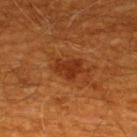Q: Was this lesion biopsied?
A: total-body-photography surveillance lesion; no biopsy
Q: Illumination type?
A: cross-polarized
Q: What is the lesion's diameter?
A: ≈3.5 mm
Q: Lesion location?
A: the upper back
Q: Who is the patient?
A: male, aged around 60
Q: What kind of image is this?
A: ~15 mm tile from a whole-body skin photo